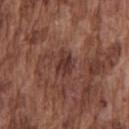The lesion was photographed on a routine skin check and not biopsied; there is no pathology result. From the chest. A lesion tile, about 15 mm wide, cut from a 3D total-body photograph. The patient is a male approximately 75 years of age.